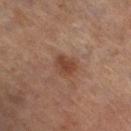Q: Was this lesion biopsied?
A: no biopsy performed (imaged during a skin exam)
Q: What is the anatomic site?
A: the left lower leg
Q: How large is the lesion?
A: ≈2.5 mm
Q: What kind of image is this?
A: total-body-photography crop, ~15 mm field of view
Q: What did automated image analysis measure?
A: an area of roughly 5 mm², an eccentricity of roughly 0.6, and a shape-asymmetry score of about 0.25 (0 = symmetric); a lesion color around L≈37 a*≈19 b*≈26 in CIELAB, roughly 8 lightness units darker than nearby skin, and a normalized lesion–skin contrast near 7.5; a border-irregularity rating of about 2/10 and a peripheral color-asymmetry measure near 1
Q: Patient demographics?
A: female, about 60 years old